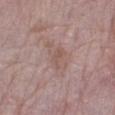• biopsy status · catalogued during a skin exam; not biopsied
• lighting · white-light illumination
• patient · female, aged 63–67
• image · 15 mm crop, total-body photography
• site · the leg
• lesion diameter · ≈2.5 mm